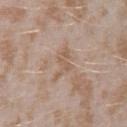biopsy status=catalogued during a skin exam; not biopsied | diameter=≈3.5 mm | patient=female, aged around 25 | illumination=white-light | site=the left forearm | imaging modality=15 mm crop, total-body photography.